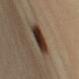Captured during whole-body skin photography for melanoma surveillance; the lesion was not biopsied.
A female patient, aged around 65.
The lesion is on the left upper arm.
A lesion tile, about 15 mm wide, cut from a 3D total-body photograph.